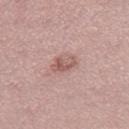The lesion was tiled from a total-body skin photograph and was not biopsied.
A female subject aged around 35.
The total-body-photography lesion software estimated a mean CIELAB color near L≈56 a*≈22 b*≈23, roughly 10 lightness units darker than nearby skin, and a normalized border contrast of about 7. The software also gave an automated nevus-likeness rating near 20 out of 100 and a detector confidence of about 100 out of 100 that the crop contains a lesion.
The recorded lesion diameter is about 3 mm.
Located on the leg.
This image is a 15 mm lesion crop taken from a total-body photograph.
The tile uses white-light illumination.